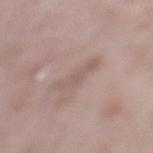No biopsy was performed on this lesion — it was imaged during a full skin examination and was not determined to be concerning. Approximately 4.5 mm at its widest. Cropped from a whole-body photographic skin survey; the tile spans about 15 mm. A female patient roughly 70 years of age. Located on the left lower leg. Imaged with white-light lighting.